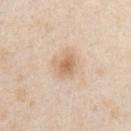lesion diameter — about 3.5 mm
automated lesion analysis — a lesion area of about 8 mm², an outline eccentricity of about 0.6 (0 = round, 1 = elongated), and two-axis asymmetry of about 0.2; a border-irregularity rating of about 2/10 and peripheral color asymmetry of about 1; a nevus-likeness score of about 75/100 and a detector confidence of about 100 out of 100 that the crop contains a lesion
body site — the chest
illumination — white-light illumination
imaging modality — ~15 mm crop, total-body skin-cancer survey
subject — male, about 45 years old